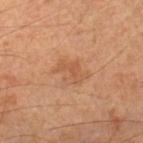{
  "biopsy_status": "not biopsied; imaged during a skin examination",
  "lesion_size": {
    "long_diameter_mm_approx": 3.5
  },
  "automated_metrics": {
    "area_mm2_approx": 6.0,
    "eccentricity": 0.75,
    "shape_asymmetry": 0.35,
    "cielab_L": 53,
    "cielab_a": 22,
    "cielab_b": 35,
    "vs_skin_darker_L": 7.0,
    "vs_skin_contrast_norm": 5.0
  },
  "site": "right lower leg",
  "image": {
    "source": "total-body photography crop",
    "field_of_view_mm": 15
  },
  "lighting": "cross-polarized",
  "patient": {
    "sex": "male",
    "age_approx": 65
  }
}